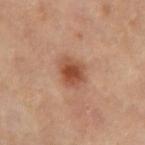Imaged during a routine full-body skin examination; the lesion was not biopsied and no histopathology is available. A female patient, in their 50s. Captured under cross-polarized illumination. A close-up tile cropped from a whole-body skin photograph, about 15 mm across. From the left thigh.